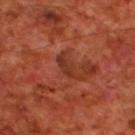Automated tile analysis of the lesion measured a border-irregularity rating of about 6.5/10, a within-lesion color-variation index near 0/10, and peripheral color asymmetry of about 0. The analysis additionally found a classifier nevus-likeness of about 0/100 and lesion-presence confidence of about 75/100. Imaged with cross-polarized lighting. From the upper back. A 15 mm close-up tile from a total-body photography series done for melanoma screening. The subject is a male approximately 70 years of age.